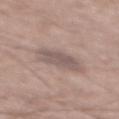| feature | finding |
|---|---|
| notes | no biopsy performed (imaged during a skin exam) |
| lighting | white-light |
| image | 15 mm crop, total-body photography |
| subject | male, aged around 60 |
| body site | the mid back |